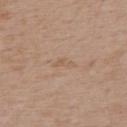| feature | finding |
|---|---|
| follow-up | total-body-photography surveillance lesion; no biopsy |
| anatomic site | the back |
| automated metrics | a lesion color around L≈57 a*≈17 b*≈31 in CIELAB, a lesion–skin lightness drop of about 5, and a normalized border contrast of about 4.5; border irregularity of about 4 on a 0–10 scale, a color-variation rating of about 0/10, and peripheral color asymmetry of about 0; a nevus-likeness score of about 0/100 |
| diameter | ~2.5 mm (longest diameter) |
| patient | male, aged around 70 |
| image | 15 mm crop, total-body photography |